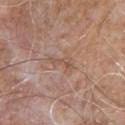The lesion was photographed on a routine skin check and not biopsied; there is no pathology result.
A 15 mm close-up extracted from a 3D total-body photography capture.
A male patient, approximately 55 years of age.
Approximately 3 mm at its widest.
The lesion is located on the front of the torso.
This is a white-light tile.